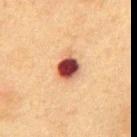Clinical impression: The lesion was photographed on a routine skin check and not biopsied; there is no pathology result. Clinical summary: The subject is a male aged approximately 75. Longest diameter approximately 3.5 mm. This is a cross-polarized tile. A 15 mm crop from a total-body photograph taken for skin-cancer surveillance. On the abdomen.